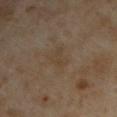Case summary:
– follow-up — catalogued during a skin exam; not biopsied
– body site — the right upper arm
– diameter — ≈3 mm
– imaging modality — ~15 mm tile from a whole-body skin photo
– lighting — cross-polarized
– subject — male, approximately 55 years of age